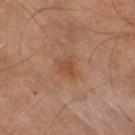Assessment:
Recorded during total-body skin imaging; not selected for excision or biopsy.
Background:
Captured under cross-polarized illumination. About 2.5 mm across. The lesion is located on the right thigh. A 15 mm close-up tile from a total-body photography series done for melanoma screening. A male subject in their 70s.